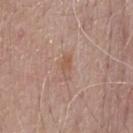This lesion was catalogued during total-body skin photography and was not selected for biopsy.
The lesion is on the chest.
The lesion-visualizer software estimated a lesion–skin lightness drop of about 7 and a lesion-to-skin contrast of about 5.5 (normalized; higher = more distinct). The analysis additionally found a border-irregularity rating of about 3.5/10. And it measured a nevus-likeness score of about 0/100 and a detector confidence of about 100 out of 100 that the crop contains a lesion.
Measured at roughly 3 mm in maximum diameter.
A male subject aged approximately 75.
A close-up tile cropped from a whole-body skin photograph, about 15 mm across.
The tile uses white-light illumination.